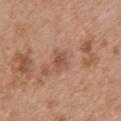Captured during whole-body skin photography for melanoma surveillance; the lesion was not biopsied.
This is a white-light tile.
On the upper back.
Approximately 2.5 mm at its widest.
A close-up tile cropped from a whole-body skin photograph, about 15 mm across.
The patient is a male aged 48 to 52.
Automated image analysis of the tile measured a footprint of about 3.5 mm², an outline eccentricity of about 0.7 (0 = round, 1 = elongated), and two-axis asymmetry of about 0.35. It also reported an average lesion color of about L≈52 a*≈24 b*≈30 (CIELAB) and roughly 9 lightness units darker than nearby skin. And it measured a border-irregularity index near 3.5/10, a color-variation rating of about 1.5/10, and peripheral color asymmetry of about 0.5. The analysis additionally found a classifier nevus-likeness of about 0/100 and a lesion-detection confidence of about 100/100.